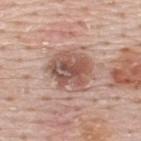• anatomic site — the back
• patient — male, about 50 years old
• tile lighting — white-light
• lesion size — about 5 mm
• imaging modality — 15 mm crop, total-body photography